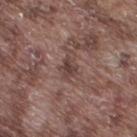<record>
<image>
  <source>total-body photography crop</source>
  <field_of_view_mm>15</field_of_view_mm>
</image>
<automated_metrics>
  <shape_asymmetry>0.3</shape_asymmetry>
  <cielab_L>41</cielab_L>
  <cielab_a>17</cielab_a>
  <cielab_b>20</cielab_b>
  <vs_skin_darker_L>9.0</vs_skin_darker_L>
  <vs_skin_contrast_norm>7.5</vs_skin_contrast_norm>
</automated_metrics>
<patient>
  <sex>male</sex>
  <age_approx>75</age_approx>
</patient>
<site>right thigh</site>
</record>